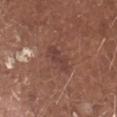The lesion was tiled from a total-body skin photograph and was not biopsied. Automated tile analysis of the lesion measured a lesion area of about 4.5 mm² and a shape eccentricity near 0.9. The software also gave a mean CIELAB color near L≈40 a*≈21 b*≈23, roughly 7 lightness units darker than nearby skin, and a lesion-to-skin contrast of about 6 (normalized; higher = more distinct). It also reported internal color variation of about 2 on a 0–10 scale and a peripheral color-asymmetry measure near 0.5. The software also gave an automated nevus-likeness rating near 5 out of 100. This is a white-light tile. From the head or neck. A 15 mm crop from a total-body photograph taken for skin-cancer surveillance. The subject is a male approximately 80 years of age.